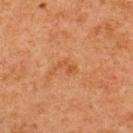follow-up — no biopsy performed (imaged during a skin exam); lighting — cross-polarized; lesion diameter — ~3 mm (longest diameter); subject — female, aged approximately 50; body site — the upper back; image source — 15 mm crop, total-body photography.